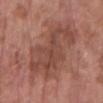Q: Was this lesion biopsied?
A: no biopsy performed (imaged during a skin exam)
Q: Who is the patient?
A: female, aged 68 to 72
Q: What is the imaging modality?
A: ~15 mm tile from a whole-body skin photo
Q: Lesion location?
A: the left forearm
Q: Illumination type?
A: white-light
Q: Automated lesion metrics?
A: a lesion color around L≈46 a*≈23 b*≈26 in CIELAB, roughly 8 lightness units darker than nearby skin, and a normalized lesion–skin contrast near 6.5
Q: Lesion size?
A: ~8.5 mm (longest diameter)